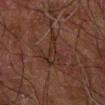Recorded during total-body skin imaging; not selected for excision or biopsy. Imaged with cross-polarized lighting. Automated image analysis of the tile measured a within-lesion color-variation index near 2/10. The software also gave a classifier nevus-likeness of about 0/100 and a detector confidence of about 80 out of 100 that the crop contains a lesion. On the right forearm. The lesion's longest dimension is about 5 mm. A 15 mm crop from a total-body photograph taken for skin-cancer surveillance. A male patient, aged approximately 70.